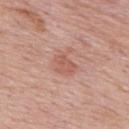Part of a total-body skin-imaging series; this lesion was reviewed on a skin check and was not flagged for biopsy.
A roughly 15 mm field-of-view crop from a total-body skin photograph.
The lesion is located on the upper back.
Imaged with white-light lighting.
A male patient, aged around 75.
An algorithmic analysis of the crop reported lesion-presence confidence of about 100/100.
Measured at roughly 3 mm in maximum diameter.